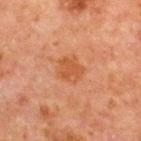| feature | finding |
|---|---|
| follow-up | total-body-photography surveillance lesion; no biopsy |
| image-analysis metrics | a nevus-likeness score of about 30/100 and lesion-presence confidence of about 100/100 |
| imaging modality | total-body-photography crop, ~15 mm field of view |
| patient | male, aged 63 to 67 |
| location | the chest |
| size | ≈3 mm |